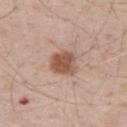The recorded lesion diameter is about 3.5 mm.
A male patient about 70 years old.
On the upper back.
A close-up tile cropped from a whole-body skin photograph, about 15 mm across.